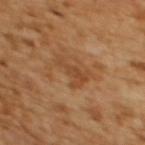Q: Was a biopsy performed?
A: catalogued during a skin exam; not biopsied
Q: How large is the lesion?
A: about 3.5 mm
Q: Lesion location?
A: the back
Q: What kind of image is this?
A: ~15 mm crop, total-body skin-cancer survey
Q: What are the patient's age and sex?
A: female, in their mid- to late 50s
Q: What did automated image analysis measure?
A: a lesion area of about 5.5 mm², an eccentricity of roughly 0.8, and a symmetry-axis asymmetry near 0.6; about 7 CIELAB-L* units darker than the surrounding skin and a normalized border contrast of about 6